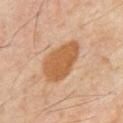<record>
  <biopsy_status>not biopsied; imaged during a skin examination</biopsy_status>
  <patient>
    <sex>male</sex>
    <age_approx>60</age_approx>
  </patient>
  <image>
    <source>total-body photography crop</source>
    <field_of_view_mm>15</field_of_view_mm>
  </image>
  <site>front of the torso</site>
</record>